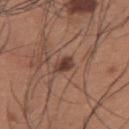{
  "biopsy_status": "not biopsied; imaged during a skin examination",
  "automated_metrics": {
    "area_mm2_approx": 3.5,
    "eccentricity": 0.75,
    "shape_asymmetry": 0.3,
    "cielab_L": 40,
    "cielab_a": 20,
    "cielab_b": 24,
    "vs_skin_darker_L": 12.0,
    "vs_skin_contrast_norm": 9.5,
    "border_irregularity_0_10": 2.5,
    "color_variation_0_10": 3.5,
    "lesion_detection_confidence_0_100": 100
  },
  "lighting": "white-light",
  "patient": {
    "sex": "male",
    "age_approx": 35
  },
  "site": "back",
  "lesion_size": {
    "long_diameter_mm_approx": 2.5
  },
  "image": {
    "source": "total-body photography crop",
    "field_of_view_mm": 15
  }
}